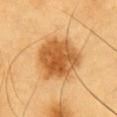Impression:
Captured during whole-body skin photography for melanoma surveillance; the lesion was not biopsied.
Image and clinical context:
This is a cross-polarized tile. The lesion is on the chest. About 4.5 mm across. This image is a 15 mm lesion crop taken from a total-body photograph. A male patient, aged approximately 60.